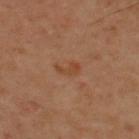Acquisition and patient details: A 15 mm close-up tile from a total-body photography series done for melanoma screening. The lesion is located on the upper back. A male patient, aged around 40.